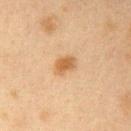biopsy status: imaged on a skin check; not biopsied | body site: the right upper arm | subject: female, approximately 40 years of age | size: ≈3 mm | automated metrics: border irregularity of about 2.5 on a 0–10 scale, a color-variation rating of about 2.5/10, and peripheral color asymmetry of about 1; a nevus-likeness score of about 95/100 | tile lighting: cross-polarized illumination | acquisition: ~15 mm crop, total-body skin-cancer survey.